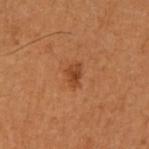Recorded during total-body skin imaging; not selected for excision or biopsy. A female subject aged 48 to 52. The lesion is on the arm. The lesion's longest dimension is about 2.5 mm. A close-up tile cropped from a whole-body skin photograph, about 15 mm across. Automated tile analysis of the lesion measured a mean CIELAB color near L≈37 a*≈23 b*≈33, roughly 8 lightness units darker than nearby skin, and a lesion-to-skin contrast of about 7 (normalized; higher = more distinct). The analysis additionally found a border-irregularity index near 2.5/10, a color-variation rating of about 2.5/10, and radial color variation of about 0.5.